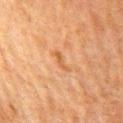Impression: The lesion was photographed on a routine skin check and not biopsied; there is no pathology result. Context: A roughly 15 mm field-of-view crop from a total-body skin photograph. Captured under cross-polarized illumination. The lesion is on the chest. A male patient aged approximately 60. About 3 mm across.